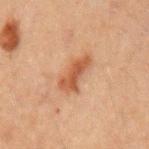patient: male, aged 48 to 52
imaging modality: ~15 mm tile from a whole-body skin photo
anatomic site: the left upper arm
lesion size: ~4.5 mm (longest diameter)
lighting: cross-polarized illumination
image-analysis metrics: internal color variation of about 2.5 on a 0–10 scale and peripheral color asymmetry of about 1